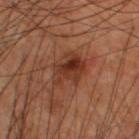workup: no biopsy performed (imaged during a skin exam) | patient: male, in their 60s | anatomic site: the back | image source: ~15 mm tile from a whole-body skin photo.